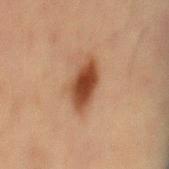{
  "biopsy_status": "not biopsied; imaged during a skin examination"
}